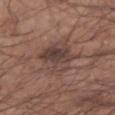Notes:
– follow-up · total-body-photography surveillance lesion; no biopsy
– lesion size · ~6 mm (longest diameter)
– tile lighting · white-light illumination
– anatomic site · the right forearm
– subject · male, in their mid- to late 50s
– acquisition · ~15 mm tile from a whole-body skin photo